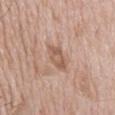Case summary:
– workup: total-body-photography surveillance lesion; no biopsy
– location: the back
– acquisition: 15 mm crop, total-body photography
– patient: male, in their mid-60s
– automated lesion analysis: a peripheral color-asymmetry measure near 0.5; a classifier nevus-likeness of about 0/100 and a lesion-detection confidence of about 100/100
– diameter: ~4 mm (longest diameter)